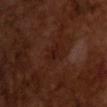follow-up: no biopsy performed (imaged during a skin exam)
lesion size: ≈3 mm
automated lesion analysis: a footprint of about 3 mm² and a shape-asymmetry score of about 0.25 (0 = symmetric); a lesion color around L≈18 a*≈21 b*≈22 in CIELAB, roughly 4 lightness units darker than nearby skin, and a lesion-to-skin contrast of about 6 (normalized; higher = more distinct); a nevus-likeness score of about 0/100 and lesion-presence confidence of about 100/100
image: 15 mm crop, total-body photography
patient: male, approximately 65 years of age